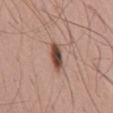Assessment: The lesion was tiled from a total-body skin photograph and was not biopsied. Image and clinical context: Cropped from a total-body skin-imaging series; the visible field is about 15 mm. This is a white-light tile. A male subject, approximately 50 years of age. From the mid back.